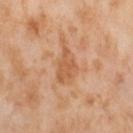Q: Was a biopsy performed?
A: total-body-photography surveillance lesion; no biopsy
Q: What is the anatomic site?
A: the left thigh
Q: What kind of image is this?
A: 15 mm crop, total-body photography
Q: Automated lesion metrics?
A: a lesion area of about 8.5 mm², an eccentricity of roughly 0.8, and two-axis asymmetry of about 0.4; a lesion color around L≈59 a*≈23 b*≈37 in CIELAB, a lesion–skin lightness drop of about 8, and a lesion-to-skin contrast of about 6 (normalized; higher = more distinct); a border-irregularity index near 4/10, internal color variation of about 2.5 on a 0–10 scale, and peripheral color asymmetry of about 1; a lesion-detection confidence of about 100/100
Q: Who is the patient?
A: female, aged 53 to 57
Q: Illumination type?
A: cross-polarized illumination
Q: What is the lesion's diameter?
A: ≈4.5 mm